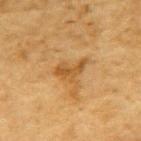The lesion was tiled from a total-body skin photograph and was not biopsied.
The lesion is located on the upper back.
A male subject, roughly 85 years of age or older.
A close-up tile cropped from a whole-body skin photograph, about 15 mm across.
This is a cross-polarized tile.
Longest diameter approximately 4 mm.
An algorithmic analysis of the crop reported a shape eccentricity near 0.85 and a shape-asymmetry score of about 0.45 (0 = symmetric). The software also gave a mean CIELAB color near L≈47 a*≈19 b*≈39, a lesion–skin lightness drop of about 8, and a normalized border contrast of about 7.